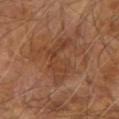A lesion tile, about 15 mm wide, cut from a 3D total-body photograph.
On the right upper arm.
A male patient, in their 70s.